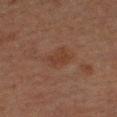{"biopsy_status": "not biopsied; imaged during a skin examination", "patient": {"sex": "male", "age_approx": 65}, "image": {"source": "total-body photography crop", "field_of_view_mm": 15}, "site": "right upper arm", "automated_metrics": {"eccentricity": 0.65, "shape_asymmetry": 0.25, "cielab_L": 30, "cielab_a": 17, "cielab_b": 23, "vs_skin_darker_L": 5.0, "vs_skin_contrast_norm": 5.5, "border_irregularity_0_10": 2.5, "color_variation_0_10": 1.5, "nevus_likeness_0_100": 5}}